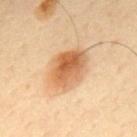Assessment:
Imaged during a routine full-body skin examination; the lesion was not biopsied and no histopathology is available.
Context:
Longest diameter approximately 6 mm. A roughly 15 mm field-of-view crop from a total-body skin photograph. The subject is a male aged around 60. On the mid back. The tile uses cross-polarized illumination.